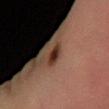Part of a total-body skin-imaging series; this lesion was reviewed on a skin check and was not flagged for biopsy.